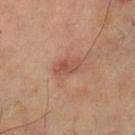workup: catalogued during a skin exam; not biopsied
image: total-body-photography crop, ~15 mm field of view
size: ≈3.5 mm
patient: female, approximately 60 years of age
illumination: cross-polarized illumination
location: the leg